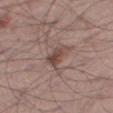Imaged during a routine full-body skin examination; the lesion was not biopsied and no histopathology is available.
A 15 mm close-up extracted from a 3D total-body photography capture.
This is a white-light tile.
The recorded lesion diameter is about 3.5 mm.
The subject is a male approximately 55 years of age.
Located on the leg.